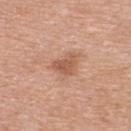Case summary:
* anatomic site: the upper back
* patient: female, roughly 40 years of age
* image: 15 mm crop, total-body photography